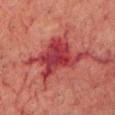Recorded during total-body skin imaging; not selected for excision or biopsy. A male patient, aged around 75. On the head or neck. A lesion tile, about 15 mm wide, cut from a 3D total-body photograph. Imaged with cross-polarized lighting.